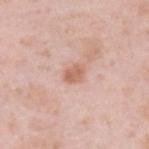workup=catalogued during a skin exam; not biopsied | image=15 mm crop, total-body photography | subject=male, aged 33–37 | anatomic site=the upper back | illumination=white-light illumination.